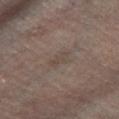Q: Was this lesion biopsied?
A: imaged on a skin check; not biopsied
Q: Lesion location?
A: the right lower leg
Q: Illumination type?
A: cross-polarized illumination
Q: How was this image acquired?
A: 15 mm crop, total-body photography
Q: Patient demographics?
A: male, aged around 55
Q: How large is the lesion?
A: ≈2.5 mm